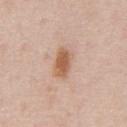| feature | finding |
|---|---|
| workup | total-body-photography surveillance lesion; no biopsy |
| size | ~3.5 mm (longest diameter) |
| tile lighting | white-light |
| automated metrics | an area of roughly 6.5 mm²; a border-irregularity rating of about 2/10, a within-lesion color-variation index near 3/10, and radial color variation of about 1 |
| image source | total-body-photography crop, ~15 mm field of view |
| site | the abdomen |
| subject | male, aged around 60 |